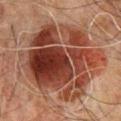Recorded during total-body skin imaging; not selected for excision or biopsy. A male subject, aged approximately 60. The recorded lesion diameter is about 10.5 mm. Automated image analysis of the tile measured a lesion color around L≈31 a*≈20 b*≈24 in CIELAB, roughly 12 lightness units darker than nearby skin, and a lesion-to-skin contrast of about 11 (normalized; higher = more distinct). The software also gave an automated nevus-likeness rating near 80 out of 100 and a lesion-detection confidence of about 100/100. The tile uses cross-polarized illumination. A region of skin cropped from a whole-body photographic capture, roughly 15 mm wide. From the chest.